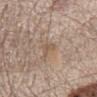Clinical impression:
Imaged during a routine full-body skin examination; the lesion was not biopsied and no histopathology is available.
Acquisition and patient details:
A 15 mm crop from a total-body photograph taken for skin-cancer surveillance. The lesion is located on the chest. Imaged with white-light lighting. A male patient, aged 68–72.